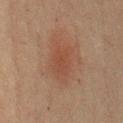Assessment:
Imaged during a routine full-body skin examination; the lesion was not biopsied and no histopathology is available.
Image and clinical context:
The tile uses cross-polarized illumination. The total-body-photography lesion software estimated a border-irregularity index near 2/10, a within-lesion color-variation index near 2/10, and radial color variation of about 1. And it measured an automated nevus-likeness rating near 80 out of 100 and a lesion-detection confidence of about 100/100. This image is a 15 mm lesion crop taken from a total-body photograph. The patient is a male aged 58–62. Located on the chest.